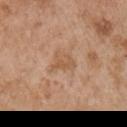Captured during whole-body skin photography for melanoma surveillance; the lesion was not biopsied.
This is a white-light tile.
The lesion is on the right upper arm.
About 3 mm across.
A region of skin cropped from a whole-body photographic capture, roughly 15 mm wide.
A male patient aged approximately 55.